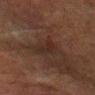workup — total-body-photography surveillance lesion; no biopsy | imaging modality — total-body-photography crop, ~15 mm field of view | subject — male, in their mid- to late 70s | automated metrics — a footprint of about 3 mm² and a shape eccentricity near 0.95; a mean CIELAB color near L≈20 a*≈15 b*≈18, about 4 CIELAB-L* units darker than the surrounding skin, and a lesion-to-skin contrast of about 6 (normalized; higher = more distinct) | site — the right forearm | illumination — cross-polarized illumination | diameter — ~3.5 mm (longest diameter).